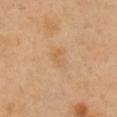Q: What is the imaging modality?
A: 15 mm crop, total-body photography
Q: Patient demographics?
A: male, in their 50s
Q: Lesion location?
A: the right upper arm